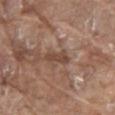workup = no biopsy performed (imaged during a skin exam).